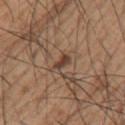{"biopsy_status": "not biopsied; imaged during a skin examination", "image": {"source": "total-body photography crop", "field_of_view_mm": 15}, "lighting": "white-light", "patient": {"sex": "male", "age_approx": 55}, "site": "left upper arm", "automated_metrics": {"cielab_L": 41, "cielab_a": 19, "cielab_b": 27, "vs_skin_darker_L": 11.0, "nevus_likeness_0_100": 85}, "lesion_size": {"long_diameter_mm_approx": 2.5}}